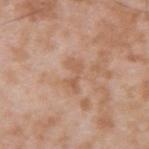Clinical impression:
Recorded during total-body skin imaging; not selected for excision or biopsy.
Image and clinical context:
A male subject, in their mid-30s. A 15 mm close-up extracted from a 3D total-body photography capture. Located on the upper back. Captured under white-light illumination. Measured at roughly 3.5 mm in maximum diameter.